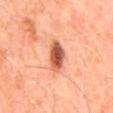biopsy_status: not biopsied; imaged during a skin examination
site: abdomen
image:
  source: total-body photography crop
  field_of_view_mm: 15
patient:
  sex: male
  age_approx: 45